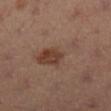Located on the leg.
A female patient, in their mid-30s.
A roughly 15 mm field-of-view crop from a total-body skin photograph.
Automated tile analysis of the lesion measured a lesion color around L≈43 a*≈17 b*≈25 in CIELAB, roughly 6 lightness units darker than nearby skin, and a normalized border contrast of about 5. And it measured a nevus-likeness score of about 80/100 and a lesion-detection confidence of about 100/100.
Longest diameter approximately 6 mm.
Captured under cross-polarized illumination.